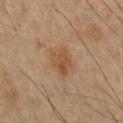No biopsy was performed on this lesion — it was imaged during a full skin examination and was not determined to be concerning. About 4 mm across. A male subject, approximately 60 years of age. A 15 mm crop from a total-body photograph taken for skin-cancer surveillance. Captured under cross-polarized illumination.